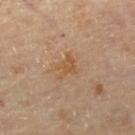  lesion_size:
    long_diameter_mm_approx: 3.0
  site: right lower leg
  image:
    source: total-body photography crop
    field_of_view_mm: 15
  lighting: cross-polarized
  patient:
    sex: female
    age_approx: 60
  automated_metrics:
    area_mm2_approx: 5.0
    eccentricity: 0.55
    shape_asymmetry: 0.4
    border_irregularity_0_10: 4.5
    color_variation_0_10: 1.5
    peripheral_color_asymmetry: 0.5
    nevus_likeness_0_100: 0